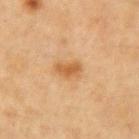* notes — imaged on a skin check; not biopsied
* imaging modality — ~15 mm tile from a whole-body skin photo
* subject — female, aged 38–42
* automated metrics — a footprint of about 5 mm², a shape eccentricity near 0.7, and a shape-asymmetry score of about 0.25 (0 = symmetric); a mean CIELAB color near L≈51 a*≈20 b*≈37, a lesion–skin lightness drop of about 9, and a lesion-to-skin contrast of about 7 (normalized; higher = more distinct); a classifier nevus-likeness of about 75/100 and a lesion-detection confidence of about 100/100
* location — the left upper arm
* lighting — cross-polarized illumination
* size — ~3 mm (longest diameter)